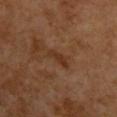| field | value |
|---|---|
| site | the upper back |
| image | total-body-photography crop, ~15 mm field of view |
| subject | male, in their 60s |
| illumination | cross-polarized |